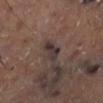follow-up — total-body-photography surveillance lesion; no biopsy
image source — 15 mm crop, total-body photography
diameter — ~3.5 mm (longest diameter)
body site — the right lower leg
image-analysis metrics — a lesion area of about 4.5 mm²; border irregularity of about 3.5 on a 0–10 scale, a color-variation rating of about 4.5/10, and radial color variation of about 1.5; a classifier nevus-likeness of about 0/100 and lesion-presence confidence of about 50/100